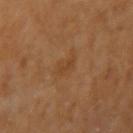The lesion was photographed on a routine skin check and not biopsied; there is no pathology result.
A 15 mm close-up tile from a total-body photography series done for melanoma screening.
The tile uses cross-polarized illumination.
From the arm.
Automated tile analysis of the lesion measured an area of roughly 3 mm² and two-axis asymmetry of about 0.35. The software also gave a classifier nevus-likeness of about 0/100 and a lesion-detection confidence of about 100/100.
A female subject aged around 50.
The lesion's longest dimension is about 3 mm.